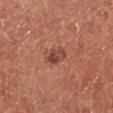workup — catalogued during a skin exam; not biopsied | body site — the right lower leg | image source — ~15 mm crop, total-body skin-cancer survey | TBP lesion metrics — border irregularity of about 3 on a 0–10 scale, a color-variation rating of about 4.5/10, and peripheral color asymmetry of about 2; an automated nevus-likeness rating near 50 out of 100 | patient — male, aged 68–72 | tile lighting — white-light.